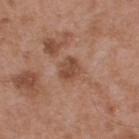Part of a total-body skin-imaging series; this lesion was reviewed on a skin check and was not flagged for biopsy.
Imaged with white-light lighting.
An algorithmic analysis of the crop reported an area of roughly 5 mm², a shape eccentricity near 0.4, and two-axis asymmetry of about 0.2. The analysis additionally found a mean CIELAB color near L≈47 a*≈22 b*≈30, about 10 CIELAB-L* units darker than the surrounding skin, and a lesion-to-skin contrast of about 7.5 (normalized; higher = more distinct). It also reported a border-irregularity rating of about 2/10 and a within-lesion color-variation index near 2.5/10. The software also gave an automated nevus-likeness rating near 0 out of 100 and a detector confidence of about 100 out of 100 that the crop contains a lesion.
The subject is a male aged around 55.
The lesion is on the upper back.
Measured at roughly 2.5 mm in maximum diameter.
Cropped from a total-body skin-imaging series; the visible field is about 15 mm.